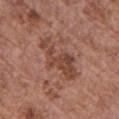  lighting: white-light
  lesion_size:
    long_diameter_mm_approx: 6.5
  site: front of the torso
  image:
    source: total-body photography crop
    field_of_view_mm: 15
  patient:
    sex: female
    age_approx: 65